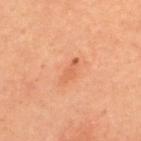lesion diameter: ~3 mm (longest diameter) | patient: female, approximately 45 years of age | site: the back | acquisition: ~15 mm crop, total-body skin-cancer survey | tile lighting: cross-polarized illumination | automated lesion analysis: an area of roughly 3 mm², a shape eccentricity near 0.9, and a shape-asymmetry score of about 0.3 (0 = symmetric); an average lesion color of about L≈62 a*≈29 b*≈40 (CIELAB), roughly 8 lightness units darker than nearby skin, and a normalized lesion–skin contrast near 5.5; a border-irregularity index near 3.5/10 and peripheral color asymmetry of about 0.